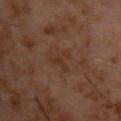Captured during whole-body skin photography for melanoma surveillance; the lesion was not biopsied.
Imaged with cross-polarized lighting.
A male subject, aged approximately 60.
Longest diameter approximately 3.5 mm.
A lesion tile, about 15 mm wide, cut from a 3D total-body photograph.
On the chest.